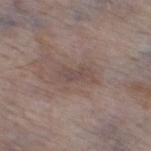Recorded during total-body skin imaging; not selected for excision or biopsy.
This is a white-light tile.
A close-up tile cropped from a whole-body skin photograph, about 15 mm across.
On the leg.
The subject is a female in their mid-80s or older.